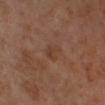Case summary:
* biopsy status: imaged on a skin check; not biopsied
* lesion size: ≈2.5 mm
* body site: the right lower leg
* acquisition: ~15 mm crop, total-body skin-cancer survey
* lighting: cross-polarized
* TBP lesion metrics: a border-irregularity index near 2.5/10, internal color variation of about 2.5 on a 0–10 scale, and a peripheral color-asymmetry measure near 1; a classifier nevus-likeness of about 0/100 and a lesion-detection confidence of about 100/100
* patient: male, approximately 70 years of age